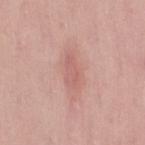biopsy status=total-body-photography surveillance lesion; no biopsy | body site=the right thigh | patient=female, aged 28 to 32 | lesion diameter=about 4 mm | illumination=white-light | image=~15 mm tile from a whole-body skin photo.